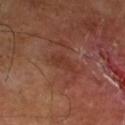Background: Captured under cross-polarized illumination. The patient is a male about 65 years old. A 15 mm close-up extracted from a 3D total-body photography capture. About 3.5 mm across.